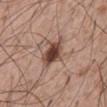{"biopsy_status": "not biopsied; imaged during a skin examination", "site": "abdomen", "automated_metrics": {"area_mm2_approx": 8.5, "eccentricity": 0.7, "shape_asymmetry": 0.3, "nevus_likeness_0_100": 95}, "lighting": "white-light", "image": {"source": "total-body photography crop", "field_of_view_mm": 15}, "patient": {"sex": "male", "age_approx": 60}}